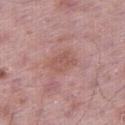Notes:
- lighting — white-light illumination
- imaging modality — ~15 mm crop, total-body skin-cancer survey
- body site — the right thigh
- subject — male, aged 48–52